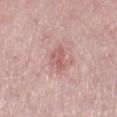Case summary:
* workup — no biopsy performed (imaged during a skin exam)
* site — the right thigh
* lighting — white-light illumination
* image — total-body-photography crop, ~15 mm field of view
* subject — male, aged around 50
* lesion size — ~3.5 mm (longest diameter)